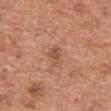Case summary:
• workup: imaged on a skin check; not biopsied
• image: ~15 mm tile from a whole-body skin photo
• patient: male, in their mid-60s
• lesion size: ≈3 mm
• tile lighting: white-light illumination
• automated metrics: a mean CIELAB color near L≈52 a*≈24 b*≈31, roughly 9 lightness units darker than nearby skin, and a normalized lesion–skin contrast near 6; border irregularity of about 4 on a 0–10 scale, a color-variation rating of about 1/10, and radial color variation of about 0.5
• anatomic site: the abdomen